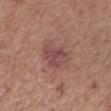  biopsy_status: not biopsied; imaged during a skin examination
  image:
    source: total-body photography crop
    field_of_view_mm: 15
  site: right forearm
  automated_metrics:
    cielab_L: 47
    cielab_a: 23
    cielab_b: 21
    vs_skin_darker_L: 8.0
    vs_skin_contrast_norm: 7.0
    border_irregularity_0_10: 3.5
    peripheral_color_asymmetry: 1.5
  lesion_size:
    long_diameter_mm_approx: 4.5
  patient:
    sex: female
    age_approx: 65
  lighting: white-light